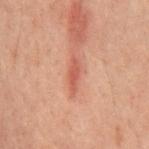- follow-up · total-body-photography surveillance lesion; no biopsy
- lesion diameter · ~3.5 mm (longest diameter)
- patient · male, aged 58–62
- anatomic site · the front of the torso
- illumination · cross-polarized
- image source · total-body-photography crop, ~15 mm field of view